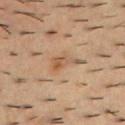patient — male, aged approximately 55
lighting — cross-polarized illumination
diameter — ~3.5 mm (longest diameter)
location — the back
image source — ~15 mm tile from a whole-body skin photo